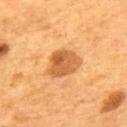Imaged during a routine full-body skin examination; the lesion was not biopsied and no histopathology is available.
Imaged with cross-polarized lighting.
Measured at roughly 4.5 mm in maximum diameter.
The lesion-visualizer software estimated a footprint of about 11 mm², an outline eccentricity of about 0.6 (0 = round, 1 = elongated), and a symmetry-axis asymmetry near 0.25. It also reported an average lesion color of about L≈55 a*≈25 b*≈43 (CIELAB).
A region of skin cropped from a whole-body photographic capture, roughly 15 mm wide.
The subject is a male approximately 55 years of age.
Located on the upper back.